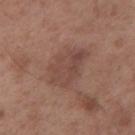automated lesion analysis = a lesion area of about 14 mm² and a shape eccentricity near 0.75
patient = male, aged 53–57
location = the mid back
tile lighting = white-light illumination
lesion diameter = ~5.5 mm (longest diameter)
image source = total-body-photography crop, ~15 mm field of view